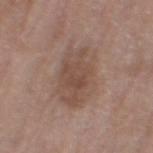Assessment: Recorded during total-body skin imaging; not selected for excision or biopsy. Context: Automated image analysis of the tile measured an area of roughly 25 mm², an outline eccentricity of about 0.75 (0 = round, 1 = elongated), and two-axis asymmetry of about 0.2. The analysis additionally found a lesion color around L≈49 a*≈17 b*≈25 in CIELAB and a lesion-to-skin contrast of about 6 (normalized; higher = more distinct). And it measured a border-irregularity rating of about 3/10 and a color-variation rating of about 3.5/10. The analysis additionally found an automated nevus-likeness rating near 0 out of 100. A region of skin cropped from a whole-body photographic capture, roughly 15 mm wide. A female subject approximately 70 years of age. The tile uses white-light illumination. Located on the left thigh.